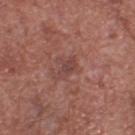- notes · catalogued during a skin exam; not biopsied